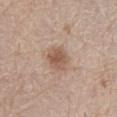Automated image analysis of the tile measured a lesion area of about 7 mm², an eccentricity of roughly 0.6, and a symmetry-axis asymmetry near 0.15.
This image is a 15 mm lesion crop taken from a total-body photograph.
A male patient, aged 78 to 82.
About 3.5 mm across.
From the abdomen.